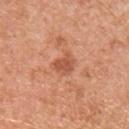| field | value |
|---|---|
| follow-up | imaged on a skin check; not biopsied |
| image source | ~15 mm tile from a whole-body skin photo |
| patient | male, in their mid- to late 50s |
| automated metrics | an area of roughly 4.5 mm², a shape eccentricity near 0.6, and two-axis asymmetry of about 0.35 |
| site | the chest |
| lesion diameter | ≈2.5 mm |
| tile lighting | white-light |